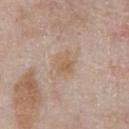Clinical impression: Imaged during a routine full-body skin examination; the lesion was not biopsied and no histopathology is available. Clinical summary: The recorded lesion diameter is about 3 mm. The lesion is on the chest. A male subject, in their mid-60s. Imaged with white-light lighting. A 15 mm close-up tile from a total-body photography series done for melanoma screening.